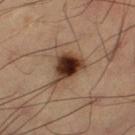{
  "biopsy_status": "not biopsied; imaged during a skin examination",
  "lighting": "cross-polarized",
  "patient": {
    "sex": "male",
    "age_approx": 60
  },
  "lesion_size": {
    "long_diameter_mm_approx": 4.5
  },
  "site": "leg",
  "image": {
    "source": "total-body photography crop",
    "field_of_view_mm": 15
  },
  "automated_metrics": {
    "area_mm2_approx": 10.0,
    "shape_asymmetry": 0.35,
    "border_irregularity_0_10": 3.5,
    "color_variation_0_10": 8.5
  }
}